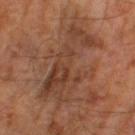The lesion was photographed on a routine skin check and not biopsied; there is no pathology result. The subject is a male in their mid- to late 60s. Automated image analysis of the tile measured an outline eccentricity of about 0.9 (0 = round, 1 = elongated) and a shape-asymmetry score of about 0.45 (0 = symmetric). It also reported a mean CIELAB color near L≈39 a*≈20 b*≈29, roughly 8 lightness units darker than nearby skin, and a normalized border contrast of about 7. And it measured an automated nevus-likeness rating near 0 out of 100 and a lesion-detection confidence of about 75/100. Imaged with cross-polarized lighting. Cropped from a whole-body photographic skin survey; the tile spans about 15 mm.